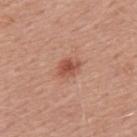lesion_size:
  long_diameter_mm_approx: 2.5
lighting: white-light
site: mid back
patient:
  sex: male
  age_approx: 50
image:
  source: total-body photography crop
  field_of_view_mm: 15
automated_metrics:
  area_mm2_approx: 4.5
  eccentricity: 0.65
  shape_asymmetry: 0.25
  cielab_L: 52
  cielab_a: 26
  cielab_b: 30
  vs_skin_darker_L: 11.0
  vs_skin_contrast_norm: 7.5
  border_irregularity_0_10: 2.5
  color_variation_0_10: 2.5
  peripheral_color_asymmetry: 0.5
  lesion_detection_confidence_0_100: 100